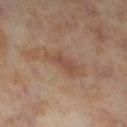This lesion was catalogued during total-body skin photography and was not selected for biopsy. The tile uses cross-polarized illumination. The subject is a female in their mid-50s. From the left lower leg. Cropped from a total-body skin-imaging series; the visible field is about 15 mm. Measured at roughly 4.5 mm in maximum diameter.